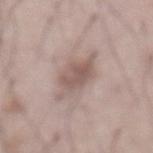Part of a total-body skin-imaging series; this lesion was reviewed on a skin check and was not flagged for biopsy.
This is a white-light tile.
The lesion is located on the abdomen.
The subject is a male approximately 55 years of age.
This image is a 15 mm lesion crop taken from a total-body photograph.
The lesion's longest dimension is about 5.5 mm.